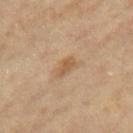The lesion is located on the leg. Automated tile analysis of the lesion measured a footprint of about 3.5 mm², an eccentricity of roughly 0.85, and two-axis asymmetry of about 0.35. And it measured an average lesion color of about L≈56 a*≈18 b*≈35 (CIELAB) and a normalized lesion–skin contrast near 6.5. A female subject, approximately 75 years of age. Approximately 3 mm at its widest. This is a cross-polarized tile. A 15 mm close-up tile from a total-body photography series done for melanoma screening.